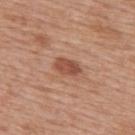Clinical impression: Captured during whole-body skin photography for melanoma surveillance; the lesion was not biopsied. Acquisition and patient details: This is a white-light tile. A roughly 15 mm field-of-view crop from a total-body skin photograph. The lesion's longest dimension is about 3.5 mm. The lesion is on the back. A male patient aged approximately 60.